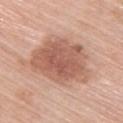Q: Is there a histopathology result?
A: total-body-photography surveillance lesion; no biopsy
Q: Patient demographics?
A: female, approximately 65 years of age
Q: What is the imaging modality?
A: 15 mm crop, total-body photography
Q: Where on the body is the lesion?
A: the upper back